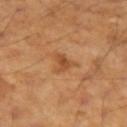Clinical impression:
The lesion was photographed on a routine skin check and not biopsied; there is no pathology result.
Image and clinical context:
The lesion's longest dimension is about 3 mm. The lesion is located on the left forearm. A roughly 15 mm field-of-view crop from a total-body skin photograph. A male subject aged approximately 45. Captured under cross-polarized illumination. Automated image analysis of the tile measured a footprint of about 4 mm², an eccentricity of roughly 0.6, and a shape-asymmetry score of about 0.5 (0 = symmetric). The analysis additionally found an average lesion color of about L≈49 a*≈24 b*≈38 (CIELAB), about 9 CIELAB-L* units darker than the surrounding skin, and a lesion-to-skin contrast of about 6.5 (normalized; higher = more distinct). The software also gave a color-variation rating of about 3/10 and a peripheral color-asymmetry measure near 1. The analysis additionally found lesion-presence confidence of about 100/100.